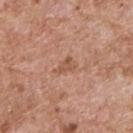notes = no biopsy performed (imaged during a skin exam) | tile lighting = white-light illumination | location = the upper back | image = total-body-photography crop, ~15 mm field of view | subject = male, aged 58–62.